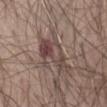{
  "biopsy_status": "not biopsied; imaged during a skin examination",
  "lighting": "white-light",
  "patient": {
    "sex": "male",
    "age_approx": 45
  },
  "image": {
    "source": "total-body photography crop",
    "field_of_view_mm": 15
  },
  "automated_metrics": {
    "area_mm2_approx": 11.0,
    "eccentricity": 0.9,
    "shape_asymmetry": 0.4
  },
  "site": "abdomen"
}